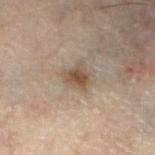– follow-up: total-body-photography surveillance lesion; no biopsy
– patient: male, about 60 years old
– imaging modality: total-body-photography crop, ~15 mm field of view
– location: the left lower leg